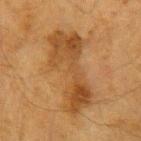| feature | finding |
|---|---|
| biopsy status | no biopsy performed (imaged during a skin exam) |
| acquisition | 15 mm crop, total-body photography |
| anatomic site | the right forearm |
| lighting | cross-polarized illumination |
| subject | female, about 55 years old |
| lesion size | ≈9 mm |